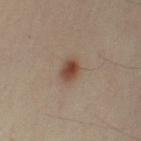Clinical impression:
The lesion was photographed on a routine skin check and not biopsied; there is no pathology result.
Context:
Imaged with cross-polarized lighting. About 2.5 mm across. From the left upper arm. A 15 mm crop from a total-body photograph taken for skin-cancer surveillance. The patient is a male aged around 50. An algorithmic analysis of the crop reported an average lesion color of about L≈40 a*≈16 b*≈24 (CIELAB) and a normalized border contrast of about 9. The analysis additionally found a border-irregularity rating of about 1.5/10, internal color variation of about 4 on a 0–10 scale, and a peripheral color-asymmetry measure near 1.